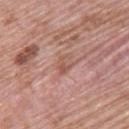The lesion is on the upper back. An algorithmic analysis of the crop reported a lesion–skin lightness drop of about 8 and a lesion-to-skin contrast of about 5.5 (normalized; higher = more distinct). The software also gave a within-lesion color-variation index near 3.5/10 and peripheral color asymmetry of about 1. A female subject, aged around 60. Cropped from a whole-body photographic skin survey; the tile spans about 15 mm.